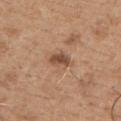Clinical impression:
No biopsy was performed on this lesion — it was imaged during a full skin examination and was not determined to be concerning.
Image and clinical context:
About 3.5 mm across. A 15 mm crop from a total-body photograph taken for skin-cancer surveillance. This is a white-light tile. Automated tile analysis of the lesion measured a lesion area of about 4.5 mm², a shape eccentricity near 0.8, and two-axis asymmetry of about 0.2. And it measured roughly 11 lightness units darker than nearby skin and a normalized border contrast of about 8. The analysis additionally found border irregularity of about 2.5 on a 0–10 scale and radial color variation of about 0.5. A male subject, aged approximately 65. On the front of the torso.